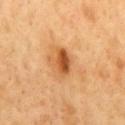workup = catalogued during a skin exam; not biopsied
imaging modality = ~15 mm crop, total-body skin-cancer survey
patient = male, roughly 50 years of age
site = the mid back